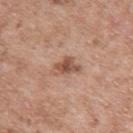Imaged during a routine full-body skin examination; the lesion was not biopsied and no histopathology is available. A male subject approximately 50 years of age. A 15 mm close-up extracted from a 3D total-body photography capture. Measured at roughly 3.5 mm in maximum diameter. From the left upper arm. The total-body-photography lesion software estimated a mean CIELAB color near L≈52 a*≈21 b*≈29 and a lesion-to-skin contrast of about 8 (normalized; higher = more distinct). The analysis additionally found a border-irregularity rating of about 4/10, internal color variation of about 4 on a 0–10 scale, and a peripheral color-asymmetry measure near 1. It also reported a classifier nevus-likeness of about 55/100. The tile uses white-light illumination.